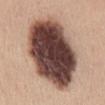Impression: Captured during whole-body skin photography for melanoma surveillance; the lesion was not biopsied. Image and clinical context: The subject is a female aged 43–47. An algorithmic analysis of the crop reported a lesion area of about 60 mm², a shape eccentricity near 0.85, and two-axis asymmetry of about 0.1. The software also gave a border-irregularity index near 2/10 and radial color variation of about 3.5. It also reported a nevus-likeness score of about 15/100. Approximately 12.5 mm at its widest. Captured under white-light illumination. A close-up tile cropped from a whole-body skin photograph, about 15 mm across. On the back.